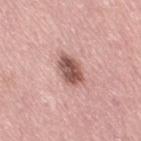Notes:
- workup: imaged on a skin check; not biopsied
- tile lighting: white-light
- lesion size: about 4 mm
- patient: female, in their mid-60s
- imaging modality: ~15 mm crop, total-body skin-cancer survey
- TBP lesion metrics: a within-lesion color-variation index near 5/10; an automated nevus-likeness rating near 75 out of 100
- body site: the right thigh